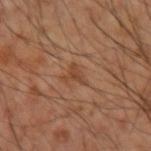biopsy status=total-body-photography surveillance lesion; no biopsy
size=≈3 mm
location=the right forearm
acquisition=total-body-photography crop, ~15 mm field of view
patient=male, aged 53–57
lighting=cross-polarized illumination